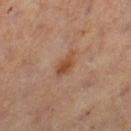<tbp_lesion>
<biopsy_status>not biopsied; imaged during a skin examination</biopsy_status>
<image>
  <source>total-body photography crop</source>
  <field_of_view_mm>15</field_of_view_mm>
</image>
<site>left thigh</site>
<patient>
  <sex>male</sex>
  <age_approx>60</age_approx>
</patient>
<lighting>cross-polarized</lighting>
<automated_metrics>
  <area_mm2_approx>4.5</area_mm2_approx>
  <shape_asymmetry>0.35</shape_asymmetry>
  <cielab_L>44</cielab_L>
  <cielab_a>21</cielab_a>
  <cielab_b>30</cielab_b>
  <vs_skin_darker_L>8.0</vs_skin_darker_L>
  <vs_skin_contrast_norm>7.5</vs_skin_contrast_norm>
  <border_irregularity_0_10>3.5</border_irregularity_0_10>
  <color_variation_0_10>2.0</color_variation_0_10>
  <peripheral_color_asymmetry>0.5</peripheral_color_asymmetry>
</automated_metrics>
</tbp_lesion>